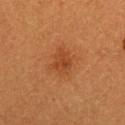Notes:
- notes — catalogued during a skin exam; not biopsied
- body site — the left upper arm
- subject — female, aged 28 to 32
- illumination — cross-polarized illumination
- image source — 15 mm crop, total-body photography